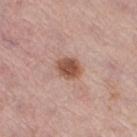The lesion was photographed on a routine skin check and not biopsied; there is no pathology result. A 15 mm crop from a total-body photograph taken for skin-cancer surveillance. Measured at roughly 3 mm in maximum diameter. The total-body-photography lesion software estimated a lesion area of about 6.5 mm², an outline eccentricity of about 0.6 (0 = round, 1 = elongated), and a shape-asymmetry score of about 0.2 (0 = symmetric). The software also gave about 13 CIELAB-L* units darker than the surrounding skin. It also reported a classifier nevus-likeness of about 95/100 and a lesion-detection confidence of about 100/100. The subject is a female aged around 45. The lesion is located on the right thigh.